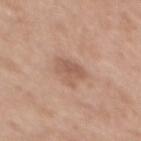Q: What did automated image analysis measure?
A: an area of roughly 6.5 mm², an eccentricity of roughly 0.65, and a symmetry-axis asymmetry near 0.4; a lesion color around L≈57 a*≈20 b*≈28 in CIELAB and about 9 CIELAB-L* units darker than the surrounding skin; a border-irregularity index near 4/10, a within-lesion color-variation index near 2.5/10, and a peripheral color-asymmetry measure near 1
Q: Where on the body is the lesion?
A: the mid back
Q: How was the tile lit?
A: white-light
Q: Patient demographics?
A: female, approximately 40 years of age
Q: What is the lesion's diameter?
A: ≈3.5 mm
Q: What kind of image is this?
A: 15 mm crop, total-body photography